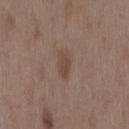Q: Was this lesion biopsied?
A: total-body-photography surveillance lesion; no biopsy
Q: Where on the body is the lesion?
A: the mid back
Q: What are the patient's age and sex?
A: female, aged 33 to 37
Q: What is the imaging modality?
A: ~15 mm tile from a whole-body skin photo